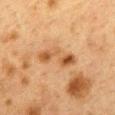- follow-up — catalogued during a skin exam; not biopsied
- automated lesion analysis — a shape eccentricity near 0.95 and two-axis asymmetry of about 0.4; a peripheral color-asymmetry measure near 2.5; a classifier nevus-likeness of about 0/100 and a lesion-detection confidence of about 100/100
- image — 15 mm crop, total-body photography
- patient — female, roughly 40 years of age
- illumination — cross-polarized illumination
- location — the mid back